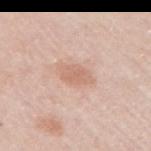This lesion was catalogued during total-body skin photography and was not selected for biopsy. Approximately 3.5 mm at its widest. A female subject, in their mid- to late 60s. From the right upper arm. The lesion-visualizer software estimated a footprint of about 6 mm², an eccentricity of roughly 0.8, and a shape-asymmetry score of about 0.2 (0 = symmetric). The software also gave a lesion-detection confidence of about 100/100. Imaged with white-light lighting. A 15 mm close-up tile from a total-body photography series done for melanoma screening.